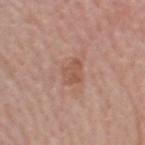Recorded during total-body skin imaging; not selected for excision or biopsy.
Automated tile analysis of the lesion measured border irregularity of about 3 on a 0–10 scale.
The lesion's longest dimension is about 3.5 mm.
Located on the head or neck.
A roughly 15 mm field-of-view crop from a total-body skin photograph.
The subject is a male roughly 50 years of age.